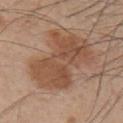notes: total-body-photography surveillance lesion; no biopsy
patient: male, aged 33 to 37
image source: ~15 mm crop, total-body skin-cancer survey
lighting: white-light
lesion size: ≈8.5 mm
body site: the upper back